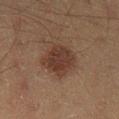Impression: Captured during whole-body skin photography for melanoma surveillance; the lesion was not biopsied. Context: The lesion-visualizer software estimated an area of roughly 14 mm², an outline eccentricity of about 0.6 (0 = round, 1 = elongated), and a symmetry-axis asymmetry near 0.2. And it measured border irregularity of about 2 on a 0–10 scale, a color-variation rating of about 3/10, and radial color variation of about 1. The lesion is on the left lower leg. A male subject, aged 43–47. Cropped from a total-body skin-imaging series; the visible field is about 15 mm. Approximately 4.5 mm at its widest.